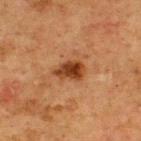{
  "patient": {
    "sex": "male",
    "age_approx": 75
  },
  "automated_metrics": {
    "area_mm2_approx": 6.5,
    "eccentricity": 0.8,
    "cielab_L": 34,
    "cielab_a": 22,
    "cielab_b": 32,
    "vs_skin_darker_L": 12.0,
    "vs_skin_contrast_norm": 10.5
  },
  "lighting": "cross-polarized",
  "image": {
    "source": "total-body photography crop",
    "field_of_view_mm": 15
  },
  "site": "upper back",
  "lesion_size": {
    "long_diameter_mm_approx": 3.5
  }
}